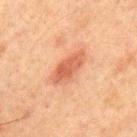Recorded during total-body skin imaging; not selected for excision or biopsy. Captured under cross-polarized illumination. Cropped from a total-body skin-imaging series; the visible field is about 15 mm. A male patient aged 63–67. Longest diameter approximately 5 mm. On the chest. Automated tile analysis of the lesion measured a within-lesion color-variation index near 3/10 and a peripheral color-asymmetry measure near 1. And it measured an automated nevus-likeness rating near 95 out of 100 and a lesion-detection confidence of about 100/100.